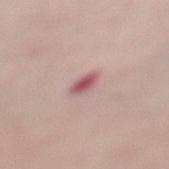<tbp_lesion>
  <biopsy_status>not biopsied; imaged during a skin examination</biopsy_status>
  <site>leg</site>
  <patient>
    <sex>female</sex>
    <age_approx>65</age_approx>
  </patient>
  <image>
    <source>total-body photography crop</source>
    <field_of_view_mm>15</field_of_view_mm>
  </image>
</tbp_lesion>